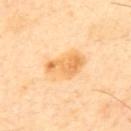The tile uses cross-polarized illumination.
A 15 mm crop from a total-body photograph taken for skin-cancer surveillance.
A male patient, in their 50s.
On the upper back.
Automated image analysis of the tile measured an area of roughly 12 mm², a shape eccentricity near 0.8, and a symmetry-axis asymmetry near 0.25. It also reported a border-irregularity rating of about 2.5/10 and internal color variation of about 5.5 on a 0–10 scale.